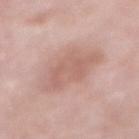  biopsy_status: not biopsied; imaged during a skin examination
  site: abdomen
  lesion_size:
    long_diameter_mm_approx: 6.0
  automated_metrics:
    area_mm2_approx: 20.0
    eccentricity: 0.75
    border_irregularity_0_10: 3.0
    color_variation_0_10: 2.0
  image:
    source: total-body photography crop
    field_of_view_mm: 15
  lighting: white-light
  patient:
    sex: male
    age_approx: 55